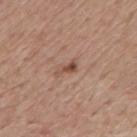Recorded during total-body skin imaging; not selected for excision or biopsy. The tile uses white-light illumination. The patient is a male in their mid-70s. Located on the mid back. A 15 mm crop from a total-body photograph taken for skin-cancer surveillance. Automated image analysis of the tile measured an area of roughly 2.5 mm², an eccentricity of roughly 0.9, and a symmetry-axis asymmetry near 0.45. The software also gave a mean CIELAB color near L≈49 a*≈21 b*≈27 and a lesion–skin lightness drop of about 11. And it measured a border-irregularity rating of about 4.5/10, internal color variation of about 0 on a 0–10 scale, and radial color variation of about 0.